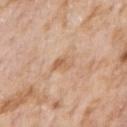Automated tile analysis of the lesion measured an average lesion color of about L≈62 a*≈20 b*≈34 (CIELAB) and roughly 8 lightness units darker than nearby skin. The software also gave an automated nevus-likeness rating near 0 out of 100 and lesion-presence confidence of about 100/100. The lesion is located on the arm. A 15 mm close-up extracted from a 3D total-body photography capture. About 3 mm across. A male subject, aged 73–77.